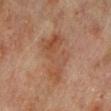The lesion was tiled from a total-body skin photograph and was not biopsied. A 15 mm crop from a total-body photograph taken for skin-cancer surveillance. A female patient, aged 78 to 82. The lesion is on the left lower leg. The tile uses cross-polarized illumination. About 6.5 mm across.